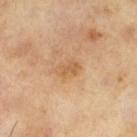  biopsy_status: not biopsied; imaged during a skin examination
  lighting: cross-polarized
  patient:
    sex: male
    age_approx: 65
  automated_metrics:
    cielab_L: 58
    cielab_a: 19
    cielab_b: 38
    vs_skin_darker_L: 7.0
    vs_skin_contrast_norm: 5.5
    nevus_likeness_0_100: 0
  lesion_size:
    long_diameter_mm_approx: 3.0
  image:
    source: total-body photography crop
    field_of_view_mm: 15